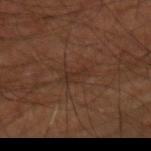{
  "biopsy_status": "not biopsied; imaged during a skin examination",
  "image": {
    "source": "total-body photography crop",
    "field_of_view_mm": 15
  },
  "lighting": "cross-polarized",
  "lesion_size": {
    "long_diameter_mm_approx": 3.5
  },
  "site": "right forearm",
  "patient": {
    "sex": "male",
    "age_approx": 70
  }
}